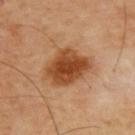Findings:
- biopsy status · no biopsy performed (imaged during a skin exam)
- diameter · ≈5.5 mm
- lighting · cross-polarized
- patient · male, aged 48 to 52
- image source · 15 mm crop, total-body photography
- anatomic site · the back